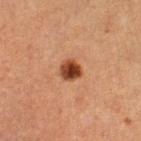Case summary:
- notes: no biopsy performed (imaged during a skin exam)
- location: the right lower leg
- imaging modality: 15 mm crop, total-body photography
- tile lighting: cross-polarized illumination
- subject: female, aged 38–42
- TBP lesion metrics: an area of roughly 5.5 mm² and an eccentricity of roughly 0.25; a detector confidence of about 100 out of 100 that the crop contains a lesion